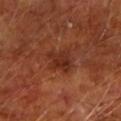Captured during whole-body skin photography for melanoma surveillance; the lesion was not biopsied. A 15 mm close-up extracted from a 3D total-body photography capture. Located on the left upper arm. A male patient, approximately 65 years of age.